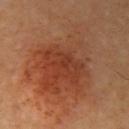Context: A 15 mm crop from a total-body photograph taken for skin-cancer surveillance. About 11 mm across. The subject is a male roughly 65 years of age. The tile uses cross-polarized illumination. The lesion is located on the right upper arm.